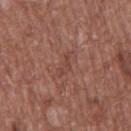Recorded during total-body skin imaging; not selected for excision or biopsy. Cropped from a total-body skin-imaging series; the visible field is about 15 mm. The lesion is on the chest. The lesion-visualizer software estimated an area of roughly 3 mm² and a shape eccentricity near 0.9. The software also gave a lesion color around L≈44 a*≈22 b*≈26 in CIELAB and a lesion-to-skin contrast of about 5 (normalized; higher = more distinct). A male patient, approximately 75 years of age.